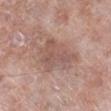A male patient, in their mid- to late 70s.
This image is a 15 mm lesion crop taken from a total-body photograph.
On the right lower leg.
The lesion-visualizer software estimated a border-irregularity rating of about 4/10, internal color variation of about 3.5 on a 0–10 scale, and peripheral color asymmetry of about 1.
Captured under white-light illumination.